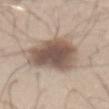Q: Patient demographics?
A: male, in their mid-40s
Q: Lesion location?
A: the abdomen
Q: How large is the lesion?
A: about 7.5 mm
Q: How was this image acquired?
A: ~15 mm tile from a whole-body skin photo
Q: What did automated image analysis measure?
A: about 15 CIELAB-L* units darker than the surrounding skin and a normalized border contrast of about 10; a nevus-likeness score of about 85/100